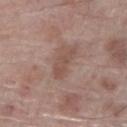Imaged during a routine full-body skin examination; the lesion was not biopsied and no histopathology is available.